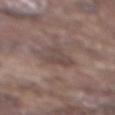This lesion was catalogued during total-body skin photography and was not selected for biopsy.
Imaged with white-light lighting.
The patient is a male approximately 75 years of age.
On the mid back.
Longest diameter approximately 4 mm.
A 15 mm close-up extracted from a 3D total-body photography capture.